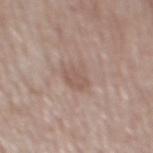Clinical impression: Recorded during total-body skin imaging; not selected for excision or biopsy. Acquisition and patient details: A male patient, in their mid- to late 50s. Automated image analysis of the tile measured a mean CIELAB color near L≈55 a*≈16 b*≈24, about 7 CIELAB-L* units darker than the surrounding skin, and a normalized lesion–skin contrast near 5. This is a white-light tile. The lesion is located on the mid back. A 15 mm crop from a total-body photograph taken for skin-cancer surveillance.